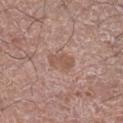workup — total-body-photography surveillance lesion; no biopsy
site — the right lower leg
patient — male, approximately 60 years of age
image source — ~15 mm tile from a whole-body skin photo
size — ≈3 mm
lighting — white-light illumination